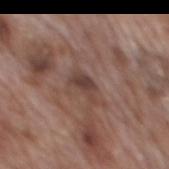Clinical impression: Captured during whole-body skin photography for melanoma surveillance; the lesion was not biopsied. Acquisition and patient details: From the mid back. Measured at roughly 3 mm in maximum diameter. A 15 mm close-up extracted from a 3D total-body photography capture. A male patient, aged 68–72. Captured under white-light illumination. Automated image analysis of the tile measured an area of roughly 4.5 mm², an outline eccentricity of about 0.85 (0 = round, 1 = elongated), and a shape-asymmetry score of about 0.45 (0 = symmetric). The software also gave a lesion-detection confidence of about 100/100.